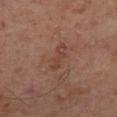Clinical impression:
Recorded during total-body skin imaging; not selected for excision or biopsy.
Background:
The tile uses cross-polarized illumination. On the left lower leg. A male subject, in their 50s. Cropped from a whole-body photographic skin survey; the tile spans about 15 mm. Longest diameter approximately 4 mm.